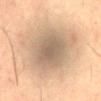Recorded during total-body skin imaging; not selected for excision or biopsy.
Measured at roughly 6 mm in maximum diameter.
The subject is a male aged 58 to 62.
The lesion is located on the abdomen.
Automated image analysis of the tile measured an average lesion color of about L≈58 a*≈12 b*≈28 (CIELAB), roughly 10 lightness units darker than nearby skin, and a lesion-to-skin contrast of about 7 (normalized; higher = more distinct). The software also gave peripheral color asymmetry of about 1. The analysis additionally found a detector confidence of about 55 out of 100 that the crop contains a lesion.
Captured under cross-polarized illumination.
A lesion tile, about 15 mm wide, cut from a 3D total-body photograph.